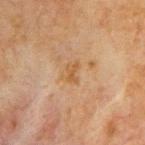biopsy_status: not biopsied; imaged during a skin examination
automated_metrics:
  eccentricity: 0.75
  cielab_L: 45
  cielab_a: 16
  cielab_b: 32
  vs_skin_darker_L: 6.0
  vs_skin_contrast_norm: 6.0
lesion_size:
  long_diameter_mm_approx: 2.5
site: chest
patient:
  sex: male
  age_approx: 65
lighting: cross-polarized
image:
  source: total-body photography crop
  field_of_view_mm: 15